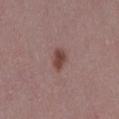  image:
    source: total-body photography crop
    field_of_view_mm: 15
  lesion_size:
    long_diameter_mm_approx: 2.5
  patient:
    sex: female
    age_approx: 45
  lighting: white-light
  automated_metrics:
    border_irregularity_0_10: 1.5
    color_variation_0_10: 2.0
    peripheral_color_asymmetry: 0.5
    lesion_detection_confidence_0_100: 100
  site: right thigh